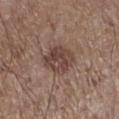biopsy_status: not biopsied; imaged during a skin examination
site: left lower leg
patient:
  sex: male
  age_approx: 60
image:
  source: total-body photography crop
  field_of_view_mm: 15
lighting: white-light